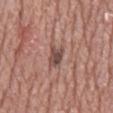Impression:
Imaged during a routine full-body skin examination; the lesion was not biopsied and no histopathology is available.
Context:
Automated image analysis of the tile measured a footprint of about 4 mm² and an eccentricity of roughly 0.85. The analysis additionally found a lesion color around L≈47 a*≈19 b*≈22 in CIELAB, about 12 CIELAB-L* units darker than the surrounding skin, and a lesion-to-skin contrast of about 8.5 (normalized; higher = more distinct). The recorded lesion diameter is about 3 mm. Captured under white-light illumination. This image is a 15 mm lesion crop taken from a total-body photograph. The patient is a male approximately 75 years of age. Located on the mid back.